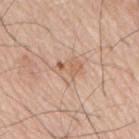Recorded during total-body skin imaging; not selected for excision or biopsy.
The lesion is on the mid back.
A male subject, roughly 80 years of age.
Approximately 3.5 mm at its widest.
The total-body-photography lesion software estimated an area of roughly 6.5 mm², an outline eccentricity of about 0.65 (0 = round, 1 = elongated), and a symmetry-axis asymmetry near 0.4. The analysis additionally found a mean CIELAB color near L≈62 a*≈19 b*≈31, a lesion–skin lightness drop of about 8, and a normalized lesion–skin contrast near 5. The analysis additionally found an automated nevus-likeness rating near 0 out of 100.
A 15 mm crop from a total-body photograph taken for skin-cancer surveillance.